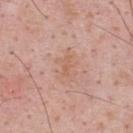Q: Was a biopsy performed?
A: total-body-photography surveillance lesion; no biopsy
Q: How was this image acquired?
A: 15 mm crop, total-body photography
Q: Where on the body is the lesion?
A: the upper back
Q: What lighting was used for the tile?
A: white-light
Q: What is the lesion's diameter?
A: ~2.5 mm (longest diameter)
Q: What did automated image analysis measure?
A: a lesion area of about 2 mm², an eccentricity of roughly 0.95, and a shape-asymmetry score of about 0.35 (0 = symmetric)
Q: What are the patient's age and sex?
A: male, in their mid- to late 50s